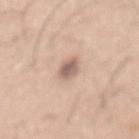  biopsy_status: not biopsied; imaged during a skin examination